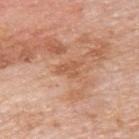biopsy status: total-body-photography surveillance lesion; no biopsy
automated lesion analysis: a lesion color around L≈57 a*≈24 b*≈34 in CIELAB, roughly 7 lightness units darker than nearby skin, and a normalized border contrast of about 5.5; a color-variation rating of about 0/10 and radial color variation of about 0; an automated nevus-likeness rating near 0 out of 100 and a lesion-detection confidence of about 100/100
subject: male, aged 73–77
illumination: white-light
image: ~15 mm crop, total-body skin-cancer survey
size: ~2.5 mm (longest diameter)
site: the upper back